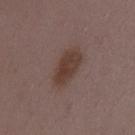Notes:
– workup: imaged on a skin check; not biopsied
– body site: the mid back
– imaging modality: ~15 mm crop, total-body skin-cancer survey
– lesion diameter: ~5.5 mm (longest diameter)
– tile lighting: white-light
– patient: female, roughly 35 years of age